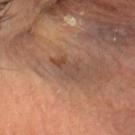Assessment:
Part of a total-body skin-imaging series; this lesion was reviewed on a skin check and was not flagged for biopsy.